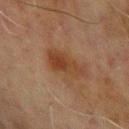Captured during whole-body skin photography for melanoma surveillance; the lesion was not biopsied. Cropped from a whole-body photographic skin survey; the tile spans about 15 mm. The lesion's longest dimension is about 4.5 mm. Located on the left forearm. A male patient, in their 70s. This is a cross-polarized tile. The total-body-photography lesion software estimated a footprint of about 9 mm², an outline eccentricity of about 0.85 (0 = round, 1 = elongated), and two-axis asymmetry of about 0.3. The software also gave a border-irregularity index near 3.5/10, internal color variation of about 4 on a 0–10 scale, and radial color variation of about 1.5.